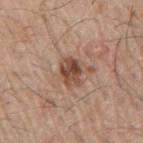Q: Was this lesion biopsied?
A: total-body-photography surveillance lesion; no biopsy
Q: What is the anatomic site?
A: the right upper arm
Q: What is the lesion's diameter?
A: ~3 mm (longest diameter)
Q: Patient demographics?
A: male, aged 63–67
Q: Illumination type?
A: white-light
Q: What kind of image is this?
A: total-body-photography crop, ~15 mm field of view